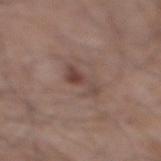notes = total-body-photography surveillance lesion; no biopsy | site = the right lower leg | tile lighting = white-light illumination | image = 15 mm crop, total-body photography | subject = male, in their mid- to late 50s | size = about 3.5 mm.